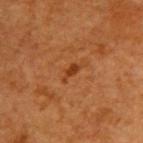Impression: Part of a total-body skin-imaging series; this lesion was reviewed on a skin check and was not flagged for biopsy. Acquisition and patient details: Imaged with cross-polarized lighting. Automated image analysis of the tile measured a lesion–skin lightness drop of about 8 and a normalized border contrast of about 7.5. It also reported a border-irregularity rating of about 4.5/10, a color-variation rating of about 0/10, and a peripheral color-asymmetry measure near 0. It also reported a nevus-likeness score of about 25/100. Located on the back. A 15 mm close-up tile from a total-body photography series done for melanoma screening. The subject is a female about 40 years old.